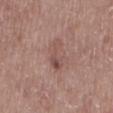Captured during whole-body skin photography for melanoma surveillance; the lesion was not biopsied. The lesion-visualizer software estimated an area of roughly 5 mm², a shape eccentricity near 0.9, and a shape-asymmetry score of about 0.55 (0 = symmetric). A male subject in their 60s. The lesion is located on the mid back. This is a white-light tile. A 15 mm crop from a total-body photograph taken for skin-cancer surveillance.